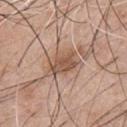Captured during whole-body skin photography for melanoma surveillance; the lesion was not biopsied. The subject is a male about 45 years old. A close-up tile cropped from a whole-body skin photograph, about 15 mm across. On the chest. The lesion's longest dimension is about 4.5 mm.